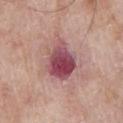Q: Was a biopsy performed?
A: total-body-photography surveillance lesion; no biopsy
Q: Patient demographics?
A: male, roughly 65 years of age
Q: Automated lesion metrics?
A: a nevus-likeness score of about 0/100 and a detector confidence of about 100 out of 100 that the crop contains a lesion
Q: What is the anatomic site?
A: the chest
Q: How was the tile lit?
A: white-light illumination
Q: How was this image acquired?
A: total-body-photography crop, ~15 mm field of view